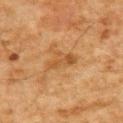follow-up: catalogued during a skin exam; not biopsied
diameter: ~4 mm (longest diameter)
lighting: cross-polarized
subject: male, aged around 80
automated metrics: a footprint of about 6 mm², an eccentricity of roughly 0.85, and a shape-asymmetry score of about 0.45 (0 = symmetric); a border-irregularity rating of about 5.5/10 and radial color variation of about 0.5
imaging modality: 15 mm crop, total-body photography
location: the upper back